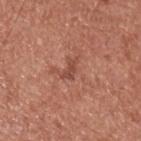Q: Was this lesion biopsied?
A: no biopsy performed (imaged during a skin exam)
Q: Where on the body is the lesion?
A: the back
Q: Automated lesion metrics?
A: a lesion color around L≈47 a*≈25 b*≈28 in CIELAB and a normalized lesion–skin contrast near 6.5; lesion-presence confidence of about 100/100
Q: How was this image acquired?
A: 15 mm crop, total-body photography
Q: Illumination type?
A: white-light
Q: Lesion size?
A: about 2.5 mm
Q: Patient demographics?
A: male, in their mid-50s